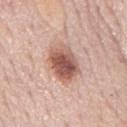Recorded during total-body skin imaging; not selected for excision or biopsy.
A 15 mm close-up extracted from a 3D total-body photography capture.
A male subject, aged 73 to 77.
Imaged with white-light lighting.
From the mid back.
Longest diameter approximately 5 mm.